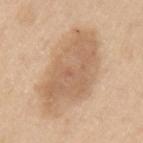follow-up — total-body-photography surveillance lesion; no biopsy | image source — total-body-photography crop, ~15 mm field of view | subject — male, in their 60s | tile lighting — white-light | automated lesion analysis — a mean CIELAB color near L≈62 a*≈17 b*≈33 and a lesion-to-skin contrast of about 6.5 (normalized; higher = more distinct); border irregularity of about 3.5 on a 0–10 scale and a color-variation rating of about 3.5/10; a detector confidence of about 100 out of 100 that the crop contains a lesion | lesion diameter — about 9.5 mm | body site — the arm.